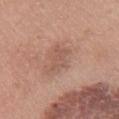{
  "lighting": "white-light",
  "automated_metrics": {
    "area_mm2_approx": 4.5,
    "eccentricity": 0.85,
    "shape_asymmetry": 0.35,
    "border_irregularity_0_10": 4.5,
    "color_variation_0_10": 1.0
  },
  "image": {
    "source": "total-body photography crop",
    "field_of_view_mm": 15
  },
  "site": "front of the torso",
  "lesion_size": {
    "long_diameter_mm_approx": 3.5
  },
  "patient": {
    "sex": "female",
    "age_approx": 60
  }
}